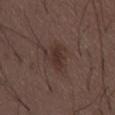Clinical impression: This lesion was catalogued during total-body skin photography and was not selected for biopsy. Context: Longest diameter approximately 3.5 mm. On the abdomen. Imaged with white-light lighting. A male subject approximately 50 years of age. An algorithmic analysis of the crop reported an area of roughly 5 mm² and a symmetry-axis asymmetry near 0.25. It also reported a mean CIELAB color near L≈31 a*≈16 b*≈19, roughly 7 lightness units darker than nearby skin, and a normalized lesion–skin contrast near 7. It also reported an automated nevus-likeness rating near 15 out of 100 and a detector confidence of about 100 out of 100 that the crop contains a lesion. A 15 mm close-up tile from a total-body photography series done for melanoma screening.